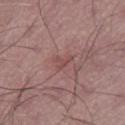  biopsy_status: not biopsied; imaged during a skin examination
  lighting: white-light
  patient:
    sex: male
    age_approx: 50
  lesion_size:
    long_diameter_mm_approx: 2.5
  site: leg
  image:
    source: total-body photography crop
    field_of_view_mm: 15